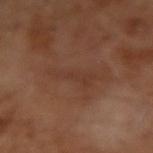| key | value |
|---|---|
| biopsy status | catalogued during a skin exam; not biopsied |
| lesion size | ≈5 mm |
| acquisition | 15 mm crop, total-body photography |
| patient | male, in their mid-60s |
| lighting | cross-polarized |